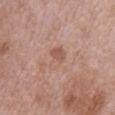imaging modality: 15 mm crop, total-body photography; illumination: white-light illumination; lesion diameter: ≈3.5 mm; patient: male, aged 48 to 52; body site: the front of the torso.